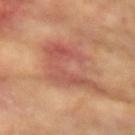Captured during whole-body skin photography for melanoma surveillance; the lesion was not biopsied.
A 15 mm close-up tile from a total-body photography series done for melanoma screening.
A female patient, roughly 75 years of age.
The lesion is on the right upper arm.